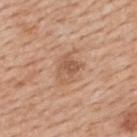lesion size: about 4 mm | location: the upper back | patient: female, about 50 years old | automated metrics: an area of roughly 7.5 mm², a shape eccentricity near 0.7, and two-axis asymmetry of about 0.3; an average lesion color of about L≈57 a*≈20 b*≈32 (CIELAB), about 9 CIELAB-L* units darker than the surrounding skin, and a lesion-to-skin contrast of about 6 (normalized; higher = more distinct) | lighting: white-light | image: ~15 mm tile from a whole-body skin photo.